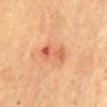Recorded during total-body skin imaging; not selected for excision or biopsy.
The lesion is on the chest.
A female patient, aged approximately 50.
Longest diameter approximately 4 mm.
A 15 mm close-up tile from a total-body photography series done for melanoma screening.